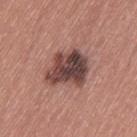Recorded during total-body skin imaging; not selected for excision or biopsy. The lesion is located on the left thigh. Imaged with white-light lighting. The patient is a female aged 58 to 62. A 15 mm crop from a total-body photograph taken for skin-cancer surveillance. About 5 mm across.